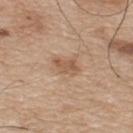Recorded during total-body skin imaging; not selected for excision or biopsy. On the back. A male patient aged around 75. Cropped from a whole-body photographic skin survey; the tile spans about 15 mm.